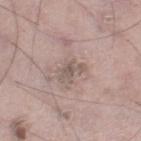The lesion was photographed on a routine skin check and not biopsied; there is no pathology result. The patient is a male approximately 65 years of age. The tile uses white-light illumination. This image is a 15 mm lesion crop taken from a total-body photograph. About 3 mm across. Located on the right thigh.